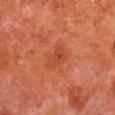notes: no biopsy performed (imaged during a skin exam) | anatomic site: the right lower leg | size: ≈3.5 mm | subject: male, aged 78 to 82 | automated lesion analysis: an average lesion color of about L≈36 a*≈28 b*≈30 (CIELAB), a lesion–skin lightness drop of about 5, and a lesion-to-skin contrast of about 4.5 (normalized; higher = more distinct) | image source: ~15 mm tile from a whole-body skin photo | lighting: cross-polarized illumination.